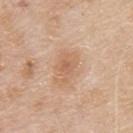  biopsy_status: not biopsied; imaged during a skin examination
  lighting: white-light
  image:
    source: total-body photography crop
    field_of_view_mm: 15
  patient:
    sex: male
    age_approx: 80
  automated_metrics:
    cielab_L: 62
    cielab_a: 20
    cielab_b: 33
    vs_skin_darker_L: 8.0
    vs_skin_contrast_norm: 5.5
    color_variation_0_10: 3.0
    peripheral_color_asymmetry: 1.0
    lesion_detection_confidence_0_100: 100
  site: upper back